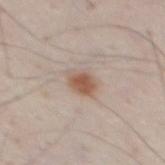{"biopsy_status": "not biopsied; imaged during a skin examination", "lighting": "white-light", "image": {"source": "total-body photography crop", "field_of_view_mm": 15}, "automated_metrics": {"cielab_L": 56, "cielab_a": 18, "cielab_b": 27, "vs_skin_darker_L": 11.0, "vs_skin_contrast_norm": 8.5, "border_irregularity_0_10": 1.5, "color_variation_0_10": 3.5, "nevus_likeness_0_100": 100, "lesion_detection_confidence_0_100": 100}, "site": "back", "patient": {"sex": "male", "age_approx": 35}}